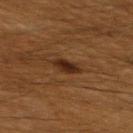Q: Is there a histopathology result?
A: no biopsy performed (imaged during a skin exam)
Q: How was this image acquired?
A: 15 mm crop, total-body photography
Q: Who is the patient?
A: male, aged 58 to 62
Q: Automated lesion metrics?
A: two-axis asymmetry of about 0.25; a mean CIELAB color near L≈25 a*≈17 b*≈27 and roughly 10 lightness units darker than nearby skin; an automated nevus-likeness rating near 90 out of 100
Q: Where on the body is the lesion?
A: the back
Q: What is the lesion's diameter?
A: ~3 mm (longest diameter)
Q: Illumination type?
A: cross-polarized